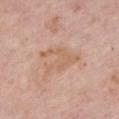Part of a total-body skin-imaging series; this lesion was reviewed on a skin check and was not flagged for biopsy. The patient is a female in their mid- to late 60s. A region of skin cropped from a whole-body photographic capture, roughly 15 mm wide. Longest diameter approximately 5 mm. Captured under white-light illumination. The lesion is on the front of the torso.